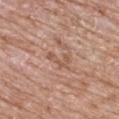The lesion was tiled from a total-body skin photograph and was not biopsied.
Approximately 3.5 mm at its widest.
An algorithmic analysis of the crop reported a footprint of about 3.5 mm², a shape eccentricity near 0.9, and two-axis asymmetry of about 0.55. It also reported an average lesion color of about L≈55 a*≈21 b*≈29 (CIELAB), about 8 CIELAB-L* units darker than the surrounding skin, and a normalized lesion–skin contrast near 6. The software also gave a border-irregularity rating of about 7/10, a color-variation rating of about 0/10, and a peripheral color-asymmetry measure near 0. And it measured a classifier nevus-likeness of about 0/100 and a lesion-detection confidence of about 80/100.
From the upper back.
A female subject in their mid-80s or older.
A 15 mm close-up tile from a total-body photography series done for melanoma screening.
The tile uses white-light illumination.